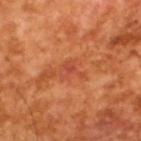biopsy_status: not biopsied; imaged during a skin examination
image:
  source: total-body photography crop
  field_of_view_mm: 15
lighting: cross-polarized
patient:
  sex: male
  age_approx: 65
automated_metrics:
  shape_asymmetry: 0.55
  cielab_L: 48
  cielab_a: 33
  cielab_b: 37
  vs_skin_darker_L: 7.0
  vs_skin_contrast_norm: 5.5
lesion_size:
  long_diameter_mm_approx: 2.5